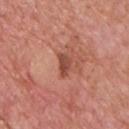No biopsy was performed on this lesion — it was imaged during a full skin examination and was not determined to be concerning.
The lesion-visualizer software estimated an area of roughly 4 mm² and a symmetry-axis asymmetry near 0.35. The analysis additionally found an average lesion color of about L≈48 a*≈27 b*≈30 (CIELAB), a lesion–skin lightness drop of about 11, and a lesion-to-skin contrast of about 8 (normalized; higher = more distinct). And it measured border irregularity of about 3.5 on a 0–10 scale and a color-variation rating of about 2/10.
On the chest.
A male patient aged 73–77.
About 3 mm across.
A region of skin cropped from a whole-body photographic capture, roughly 15 mm wide.
Imaged with white-light lighting.